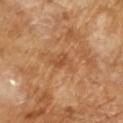This image is a 15 mm lesion crop taken from a total-body photograph. A male subject in their mid- to late 60s. An algorithmic analysis of the crop reported a footprint of about 4.5 mm² and a shape-asymmetry score of about 0.35 (0 = symmetric). And it measured a within-lesion color-variation index near 2/10 and radial color variation of about 0.5. Imaged with cross-polarized lighting. Approximately 3 mm at its widest.